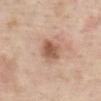notes: imaged on a skin check; not biopsied
tile lighting: white-light
acquisition: total-body-photography crop, ~15 mm field of view
subject: female, in their mid-60s
lesion size: about 3.5 mm
site: the abdomen